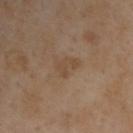Q: Was a biopsy performed?
A: total-body-photography surveillance lesion; no biopsy
Q: Patient demographics?
A: male, aged around 55
Q: How was the tile lit?
A: cross-polarized illumination
Q: What is the anatomic site?
A: the upper back
Q: How large is the lesion?
A: ~3 mm (longest diameter)
Q: Automated lesion metrics?
A: an area of roughly 4.5 mm² and two-axis asymmetry of about 0.45; border irregularity of about 5.5 on a 0–10 scale
Q: How was this image acquired?
A: 15 mm crop, total-body photography